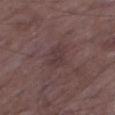{"biopsy_status": "not biopsied; imaged during a skin examination", "automated_metrics": {"area_mm2_approx": 4.0, "eccentricity": 0.75, "shape_asymmetry": 0.25}, "lesion_size": {"long_diameter_mm_approx": 2.5}, "lighting": "white-light", "site": "right thigh", "image": {"source": "total-body photography crop", "field_of_view_mm": 15}, "patient": {"sex": "male", "age_approx": 65}}